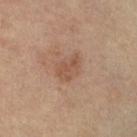Q: What lighting was used for the tile?
A: cross-polarized illumination
Q: Lesion location?
A: the right lower leg
Q: How large is the lesion?
A: about 3.5 mm
Q: Who is the patient?
A: female, in their mid- to late 60s
Q: What kind of image is this?
A: ~15 mm tile from a whole-body skin photo